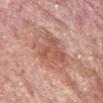Recorded during total-body skin imaging; not selected for excision or biopsy.
A lesion tile, about 15 mm wide, cut from a 3D total-body photograph.
A male subject about 60 years old.
The lesion is located on the head or neck.
Measured at roughly 8.5 mm in maximum diameter.